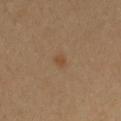<record>
  <biopsy_status>not biopsied; imaged during a skin examination</biopsy_status>
  <image>
    <source>total-body photography crop</source>
    <field_of_view_mm>15</field_of_view_mm>
  </image>
  <lesion_size>
    <long_diameter_mm_approx>1.5</long_diameter_mm_approx>
  </lesion_size>
  <patient>
    <sex>male</sex>
    <age_approx>30</age_approx>
  </patient>
  <site>arm</site>
  <automated_metrics>
    <area_mm2_approx>1.5</area_mm2_approx>
    <eccentricity>0.7</eccentricity>
    <shape_asymmetry>0.35</shape_asymmetry>
    <peripheral_color_asymmetry>0.0</peripheral_color_asymmetry>
    <nevus_likeness_0_100>75</nevus_likeness_0_100>
    <lesion_detection_confidence_0_100>100</lesion_detection_confidence_0_100>
  </automated_metrics>
</record>